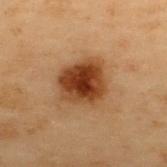workup: no biopsy performed (imaged during a skin exam)
patient: male, aged approximately 55
automated metrics: a footprint of about 17 mm², a shape eccentricity near 0.35, and a shape-asymmetry score of about 0.2 (0 = symmetric); about 14 CIELAB-L* units darker than the surrounding skin and a lesion-to-skin contrast of about 12 (normalized; higher = more distinct); a border-irregularity rating of about 2/10, a within-lesion color-variation index near 6/10, and peripheral color asymmetry of about 1.5
image: 15 mm crop, total-body photography
body site: the upper back
lesion size: ≈4.5 mm